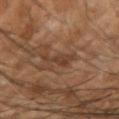Recorded during total-body skin imaging; not selected for excision or biopsy.
A male patient in their mid- to late 50s.
Automated tile analysis of the lesion measured a footprint of about 4 mm², an eccentricity of roughly 0.85, and a symmetry-axis asymmetry near 0.6. The software also gave a lesion color around L≈36 a*≈18 b*≈26 in CIELAB, about 7 CIELAB-L* units darker than the surrounding skin, and a normalized border contrast of about 6. The analysis additionally found border irregularity of about 7.5 on a 0–10 scale, a within-lesion color-variation index near 1.5/10, and a peripheral color-asymmetry measure near 0.5.
Approximately 3 mm at its widest.
From the right forearm.
A 15 mm close-up extracted from a 3D total-body photography capture.